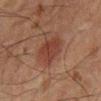<record>
<biopsy_status>not biopsied; imaged during a skin examination</biopsy_status>
<image>
  <source>total-body photography crop</source>
  <field_of_view_mm>15</field_of_view_mm>
</image>
<lighting>cross-polarized</lighting>
<lesion_size>
  <long_diameter_mm_approx>4.0</long_diameter_mm_approx>
</lesion_size>
<site>abdomen</site>
<patient>
  <sex>male</sex>
  <age_approx>80</age_approx>
</patient>
<automated_metrics>
  <vs_skin_darker_L>7.0</vs_skin_darker_L>
  <border_irregularity_0_10>2.5</border_irregularity_0_10>
  <color_variation_0_10>2.5</color_variation_0_10>
  <nevus_likeness_0_100>75</nevus_likeness_0_100>
  <lesion_detection_confidence_0_100>100</lesion_detection_confidence_0_100>
</automated_metrics>
</record>